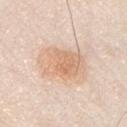<tbp_lesion>
<biopsy_status>not biopsied; imaged during a skin examination</biopsy_status>
<image>
  <source>total-body photography crop</source>
  <field_of_view_mm>15</field_of_view_mm>
</image>
<site>chest</site>
<patient>
  <sex>male</sex>
  <age_approx>35</age_approx>
</patient>
<lighting>white-light</lighting>
<lesion_size>
  <long_diameter_mm_approx>5.5</long_diameter_mm_approx>
</lesion_size>
<automated_metrics>
  <eccentricity>0.7</eccentricity>
  <shape_asymmetry>0.15</shape_asymmetry>
  <nevus_likeness_0_100>85</nevus_likeness_0_100>
  <lesion_detection_confidence_0_100>100</lesion_detection_confidence_0_100>
</automated_metrics>
</tbp_lesion>